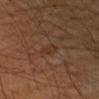notes — total-body-photography surveillance lesion; no biopsy | patient — male, aged 58–62 | image source — ~15 mm tile from a whole-body skin photo | illumination — cross-polarized | location — the arm | size — about 3 mm | TBP lesion metrics — an area of roughly 3.5 mm², an outline eccentricity of about 0.85 (0 = round, 1 = elongated), and a symmetry-axis asymmetry near 0.3; an average lesion color of about L≈30 a*≈16 b*≈26 (CIELAB), roughly 4 lightness units darker than nearby skin, and a normalized border contrast of about 5; a classifier nevus-likeness of about 0/100 and a lesion-detection confidence of about 100/100.